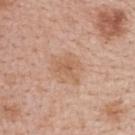Clinical impression:
Recorded during total-body skin imaging; not selected for excision or biopsy.
Background:
The lesion is located on the upper back. A 15 mm close-up extracted from a 3D total-body photography capture. A female subject aged approximately 50.